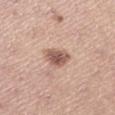* biopsy status: total-body-photography surveillance lesion; no biopsy
* acquisition: 15 mm crop, total-body photography
* diameter: ~3.5 mm (longest diameter)
* patient: female, in their mid- to late 20s
* anatomic site: the right lower leg
* automated metrics: a classifier nevus-likeness of about 80/100 and lesion-presence confidence of about 100/100